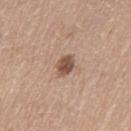workup: catalogued during a skin exam; not biopsied
subject: female, about 70 years old
size: ~2.5 mm (longest diameter)
illumination: white-light illumination
image: ~15 mm crop, total-body skin-cancer survey
body site: the left thigh